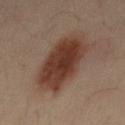Recorded during total-body skin imaging; not selected for excision or biopsy. Captured under cross-polarized illumination. A male subject, aged 48–52. This image is a 15 mm lesion crop taken from a total-body photograph. Measured at roughly 8.5 mm in maximum diameter. On the mid back.